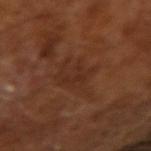  biopsy_status: not biopsied; imaged during a skin examination
  automated_metrics:
    border_irregularity_0_10: 5.5
    color_variation_0_10: 2.5
    peripheral_color_asymmetry: 0.5
    nevus_likeness_0_100: 0
    lesion_detection_confidence_0_100: 85
  lighting: cross-polarized
  lesion_size:
    long_diameter_mm_approx: 5.0
  patient:
    sex: male
    age_approx: 65
  image:
    source: total-body photography crop
    field_of_view_mm: 15